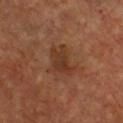The lesion is located on the chest.
Approximately 4.5 mm at its widest.
The subject is a male about 50 years old.
A region of skin cropped from a whole-body photographic capture, roughly 15 mm wide.
Imaged with cross-polarized lighting.